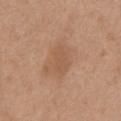No biopsy was performed on this lesion — it was imaged during a full skin examination and was not determined to be concerning. A 15 mm close-up extracted from a 3D total-body photography capture. About 3.5 mm across. Automated tile analysis of the lesion measured a lesion color around L≈54 a*≈20 b*≈32 in CIELAB, about 6 CIELAB-L* units darker than the surrounding skin, and a normalized border contrast of about 4.5. The tile uses white-light illumination. The lesion is on the chest. A male patient in their 70s.